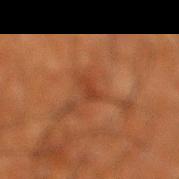* biopsy status: total-body-photography surveillance lesion; no biopsy
* automated lesion analysis: a lesion area of about 3 mm² and an eccentricity of roughly 0.85; an average lesion color of about L≈32 a*≈23 b*≈30 (CIELAB), a lesion–skin lightness drop of about 6, and a normalized border contrast of about 5.5; an automated nevus-likeness rating near 0 out of 100 and lesion-presence confidence of about 85/100
* subject: male, aged approximately 65
* acquisition: total-body-photography crop, ~15 mm field of view
* site: the right lower leg
* lesion diameter: ~2.5 mm (longest diameter)